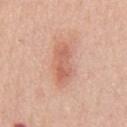notes: imaged on a skin check; not biopsied
lesion diameter: ~4.5 mm (longest diameter)
location: the chest
patient: male, about 50 years old
image-analysis metrics: a lesion area of about 7.5 mm², an outline eccentricity of about 0.9 (0 = round, 1 = elongated), and a symmetry-axis asymmetry near 0.25; a mean CIELAB color near L≈62 a*≈25 b*≈31 and a lesion-to-skin contrast of about 6.5 (normalized; higher = more distinct)
lighting: white-light
acquisition: ~15 mm crop, total-body skin-cancer survey